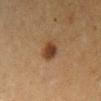| key | value |
|---|---|
| follow-up | imaged on a skin check; not biopsied |